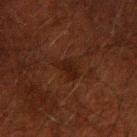Imaged during a routine full-body skin examination; the lesion was not biopsied and no histopathology is available.
A lesion tile, about 15 mm wide, cut from a 3D total-body photograph.
Captured under cross-polarized illumination.
A male patient approximately 50 years of age.
The lesion is on the right upper arm.
The lesion's longest dimension is about 3 mm.